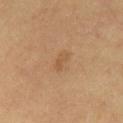Findings:
• workup: no biopsy performed (imaged during a skin exam)
• automated metrics: a shape-asymmetry score of about 0.3 (0 = symmetric); an automated nevus-likeness rating near 0 out of 100 and a detector confidence of about 100 out of 100 that the crop contains a lesion
• image: 15 mm crop, total-body photography
• subject: male, aged 58 to 62
• diameter: about 2.5 mm
• body site: the front of the torso